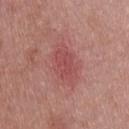This lesion was catalogued during total-body skin photography and was not selected for biopsy. Cropped from a whole-body photographic skin survey; the tile spans about 15 mm. The subject is a male aged 28–32. Approximately 4.5 mm at its widest.